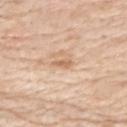notes: imaged on a skin check; not biopsied | location: the mid back | imaging modality: ~15 mm tile from a whole-body skin photo | tile lighting: white-light | patient: male, in their mid- to late 70s | image-analysis metrics: a lesion area of about 2.5 mm², an eccentricity of roughly 0.95, and a shape-asymmetry score of about 0.45 (0 = symmetric); a lesion color around L≈65 a*≈19 b*≈34 in CIELAB, roughly 10 lightness units darker than nearby skin, and a lesion-to-skin contrast of about 7 (normalized; higher = more distinct); border irregularity of about 4 on a 0–10 scale and peripheral color asymmetry of about 0; a nevus-likeness score of about 0/100 and lesion-presence confidence of about 100/100.